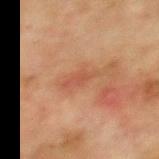  biopsy_status: not biopsied; imaged during a skin examination
  site: mid back
  lighting: cross-polarized
  image:
    source: total-body photography crop
    field_of_view_mm: 15
  patient:
    sex: male
    age_approx: 75
  automated_metrics:
    lesion_detection_confidence_0_100: 100